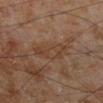A male subject, approximately 70 years of age. Located on the left lower leg. The lesion-visualizer software estimated an average lesion color of about L≈39 a*≈17 b*≈28 (CIELAB), roughly 5 lightness units darker than nearby skin, and a normalized border contrast of about 5. The software also gave a nevus-likeness score of about 0/100 and lesion-presence confidence of about 95/100. A region of skin cropped from a whole-body photographic capture, roughly 15 mm wide. The tile uses cross-polarized illumination. Approximately 5 mm at its widest.